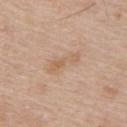notes: imaged on a skin check; not biopsied | site: the upper back | imaging modality: ~15 mm crop, total-body skin-cancer survey | patient: male, in their 80s.